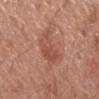Impression:
No biopsy was performed on this lesion — it was imaged during a full skin examination and was not determined to be concerning.
Background:
Captured under white-light illumination. Approximately 4.5 mm at its widest. A female patient about 50 years old. A close-up tile cropped from a whole-body skin photograph, about 15 mm across. The lesion is located on the left forearm.